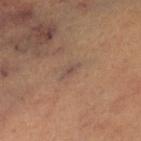Notes:
• follow-up: no biopsy performed (imaged during a skin exam)
• size: ≈2.5 mm
• image source: ~15 mm crop, total-body skin-cancer survey
• subject: female, aged around 45
• site: the right leg
• tile lighting: cross-polarized
• image-analysis metrics: a lesion area of about 2 mm² and a shape eccentricity near 0.9; a mean CIELAB color near L≈47 a*≈16 b*≈23 and roughly 6 lightness units darker than nearby skin; a border-irregularity index near 3.5/10, a within-lesion color-variation index near 0/10, and a peripheral color-asymmetry measure near 0